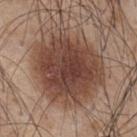biopsy status=no biopsy performed (imaged during a skin exam)
lighting=white-light illumination
body site=the back
imaging modality=15 mm crop, total-body photography
size=~9.5 mm (longest diameter)
subject=male, aged around 45